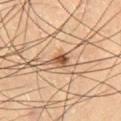The lesion was photographed on a routine skin check and not biopsied; there is no pathology result. The lesion is on the right thigh. The patient is a male in their mid- to late 60s. A close-up tile cropped from a whole-body skin photograph, about 15 mm across.